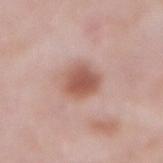Part of a total-body skin-imaging series; this lesion was reviewed on a skin check and was not flagged for biopsy. The patient is a male aged 53–57. A 15 mm close-up tile from a total-body photography series done for melanoma screening. On the abdomen.